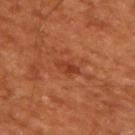notes — total-body-photography surveillance lesion; no biopsy
location — the upper back
automated metrics — a lesion area of about 4 mm², a shape eccentricity near 0.8, and a symmetry-axis asymmetry near 0.35; an average lesion color of about L≈39 a*≈29 b*≈34 (CIELAB), a lesion–skin lightness drop of about 7, and a normalized lesion–skin contrast near 6; an automated nevus-likeness rating near 15 out of 100 and a detector confidence of about 100 out of 100 that the crop contains a lesion
patient — male, aged 63–67
image source — ~15 mm crop, total-body skin-cancer survey
size — ≈3 mm
illumination — cross-polarized illumination